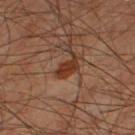Case summary:
– follow-up · catalogued during a skin exam; not biopsied
– body site · the left thigh
– image · total-body-photography crop, ~15 mm field of view
– tile lighting · cross-polarized
– subject · male, aged 58–62
– size · ~3.5 mm (longest diameter)